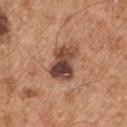Recorded during total-body skin imaging; not selected for excision or biopsy. The patient is a male approximately 55 years of age. The lesion-visualizer software estimated a lesion area of about 11 mm², a shape eccentricity near 0.75, and two-axis asymmetry of about 0.25. The software also gave roughly 15 lightness units darker than nearby skin. The analysis additionally found a border-irregularity index near 3/10, a within-lesion color-variation index near 10/10, and radial color variation of about 3.5. The analysis additionally found a nevus-likeness score of about 10/100 and lesion-presence confidence of about 100/100. This is a white-light tile. A roughly 15 mm field-of-view crop from a total-body skin photograph. On the right upper arm.